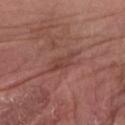Q: Was a biopsy performed?
A: no biopsy performed (imaged during a skin exam)
Q: Patient demographics?
A: male, roughly 80 years of age
Q: Automated lesion metrics?
A: a lesion area of about 5 mm², an outline eccentricity of about 0.9 (0 = round, 1 = elongated), and a shape-asymmetry score of about 0.35 (0 = symmetric); an average lesion color of about L≈43 a*≈23 b*≈24 (CIELAB), roughly 7 lightness units darker than nearby skin, and a normalized lesion–skin contrast near 5.5; border irregularity of about 4 on a 0–10 scale, a within-lesion color-variation index near 2.5/10, and radial color variation of about 1; a classifier nevus-likeness of about 0/100 and a detector confidence of about 80 out of 100 that the crop contains a lesion
Q: Where on the body is the lesion?
A: the right forearm
Q: Illumination type?
A: white-light illumination
Q: What is the imaging modality?
A: 15 mm crop, total-body photography
Q: Lesion size?
A: ~4 mm (longest diameter)